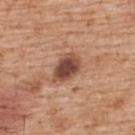Q: Was a biopsy performed?
A: no biopsy performed (imaged during a skin exam)
Q: What is the anatomic site?
A: the back
Q: How was the tile lit?
A: white-light
Q: What is the imaging modality?
A: 15 mm crop, total-body photography
Q: Automated lesion metrics?
A: a footprint of about 8.5 mm² and a symmetry-axis asymmetry near 0.25; a lesion color around L≈47 a*≈22 b*≈28 in CIELAB; a border-irregularity index near 2.5/10, a color-variation rating of about 4.5/10, and peripheral color asymmetry of about 1.5
Q: Patient demographics?
A: male, approximately 60 years of age